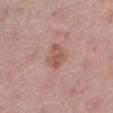{"biopsy_status": "not biopsied; imaged during a skin examination", "site": "left lower leg", "automated_metrics": {"area_mm2_approx": 6.0, "eccentricity": 0.7, "cielab_L": 55, "cielab_a": 22, "cielab_b": 26, "vs_skin_darker_L": 9.0, "border_irregularity_0_10": 2.5, "peripheral_color_asymmetry": 1.0}, "image": {"source": "total-body photography crop", "field_of_view_mm": 15}, "lesion_size": {"long_diameter_mm_approx": 3.0}, "patient": {"sex": "male", "age_approx": 50}}